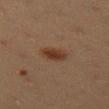biopsy status: total-body-photography surveillance lesion; no biopsy | image: total-body-photography crop, ~15 mm field of view | patient: female, aged 18 to 22 | anatomic site: the left thigh | lighting: cross-polarized | TBP lesion metrics: an area of roughly 5 mm², an outline eccentricity of about 0.8 (0 = round, 1 = elongated), and two-axis asymmetry of about 0.2; a lesion color around L≈31 a*≈18 b*≈26 in CIELAB; a lesion-detection confidence of about 100/100.